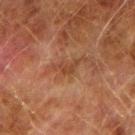biopsy status: catalogued during a skin exam; not biopsied
imaging modality: ~15 mm crop, total-body skin-cancer survey
subject: male, aged 73 to 77
location: the right upper arm
TBP lesion metrics: an area of roughly 3.5 mm², an outline eccentricity of about 0.9 (0 = round, 1 = elongated), and a shape-asymmetry score of about 0.55 (0 = symmetric); a nevus-likeness score of about 0/100